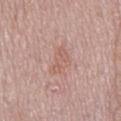biopsy_status: not biopsied; imaged during a skin examination
image:
  source: total-body photography crop
  field_of_view_mm: 15
lighting: white-light
site: mid back
patient:
  sex: male
  age_approx: 75
lesion_size:
  long_diameter_mm_approx: 3.5
automated_metrics:
  cielab_L: 59
  cielab_a: 21
  cielab_b: 25
  vs_skin_darker_L: 6.0
  vs_skin_contrast_norm: 4.5